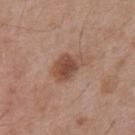follow-up = imaged on a skin check; not biopsied
subject = male, aged 53 to 57
location = the mid back
image source = 15 mm crop, total-body photography